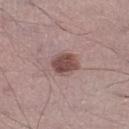The lesion was photographed on a routine skin check and not biopsied; there is no pathology result. The lesion is on the right lower leg. The patient is a male aged around 60. Longest diameter approximately 3 mm. A 15 mm crop from a total-body photograph taken for skin-cancer surveillance.